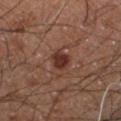Assessment:
Imaged during a routine full-body skin examination; the lesion was not biopsied and no histopathology is available.
Image and clinical context:
The recorded lesion diameter is about 3 mm. The patient is a male aged 58–62. Automated tile analysis of the lesion measured a lesion area of about 5 mm², a shape eccentricity near 0.7, and two-axis asymmetry of about 0.25. It also reported a lesion color around L≈29 a*≈20 b*≈23 in CIELAB, a lesion–skin lightness drop of about 10, and a normalized lesion–skin contrast near 9.5. It also reported internal color variation of about 2.5 on a 0–10 scale and radial color variation of about 1. Captured under cross-polarized illumination. The lesion is located on the right thigh. Cropped from a whole-body photographic skin survey; the tile spans about 15 mm.